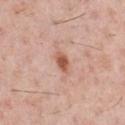Clinical summary:
A male subject aged approximately 50. Automated tile analysis of the lesion measured a footprint of about 3.5 mm² and an eccentricity of roughly 0.85. The software also gave an average lesion color of about L≈57 a*≈24 b*≈31 (CIELAB), about 13 CIELAB-L* units darker than the surrounding skin, and a lesion-to-skin contrast of about 9 (normalized; higher = more distinct). On the front of the torso. A 15 mm close-up extracted from a 3D total-body photography capture. Imaged with white-light lighting. The recorded lesion diameter is about 3 mm.